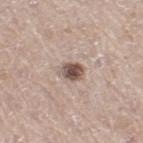follow-up: no biopsy performed (imaged during a skin exam) | automated metrics: a lesion area of about 5.5 mm², a shape eccentricity near 0.65, and two-axis asymmetry of about 0.15; a lesion–skin lightness drop of about 15 and a normalized border contrast of about 10; a color-variation rating of about 6/10 and radial color variation of about 2; a nevus-likeness score of about 95/100 and a detector confidence of about 100 out of 100 that the crop contains a lesion | tile lighting: white-light illumination | imaging modality: total-body-photography crop, ~15 mm field of view | body site: the right thigh | size: ~3 mm (longest diameter) | patient: male, in their mid- to late 60s.